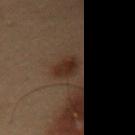Clinical impression: Part of a total-body skin-imaging series; this lesion was reviewed on a skin check and was not flagged for biopsy. Background: The subject is a male aged 53–57. On the abdomen. This is a cross-polarized tile. A lesion tile, about 15 mm wide, cut from a 3D total-body photograph. Automated image analysis of the tile measured border irregularity of about 2 on a 0–10 scale and a within-lesion color-variation index near 2.5/10. The analysis additionally found an automated nevus-likeness rating near 85 out of 100 and a detector confidence of about 100 out of 100 that the crop contains a lesion.